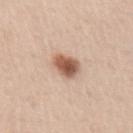Captured during whole-body skin photography for melanoma surveillance; the lesion was not biopsied. On the left upper arm. Longest diameter approximately 3 mm. Imaged with white-light lighting. A male patient, aged around 60. A lesion tile, about 15 mm wide, cut from a 3D total-body photograph.